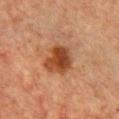No biopsy was performed on this lesion — it was imaged during a full skin examination and was not determined to be concerning.